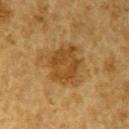This lesion was catalogued during total-body skin photography and was not selected for biopsy.
This is a cross-polarized tile.
A male patient aged around 85 or older.
Automated tile analysis of the lesion measured a border-irregularity rating of about 4/10, a color-variation rating of about 3.5/10, and radial color variation of about 1. The analysis additionally found a classifier nevus-likeness of about 60/100 and a lesion-detection confidence of about 100/100.
The lesion is on the arm.
This image is a 15 mm lesion crop taken from a total-body photograph.